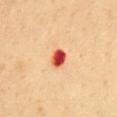Q: Is there a histopathology result?
A: total-body-photography surveillance lesion; no biopsy
Q: Who is the patient?
A: female, roughly 50 years of age
Q: How large is the lesion?
A: ≈2.5 mm
Q: Where on the body is the lesion?
A: the chest
Q: What lighting was used for the tile?
A: cross-polarized
Q: What is the imaging modality?
A: total-body-photography crop, ~15 mm field of view